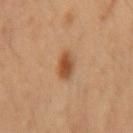Recorded during total-body skin imaging; not selected for excision or biopsy.
A lesion tile, about 15 mm wide, cut from a 3D total-body photograph.
The lesion is located on the right forearm.
Measured at roughly 2.5 mm in maximum diameter.
A female patient, aged around 35.